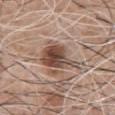Impression: Part of a total-body skin-imaging series; this lesion was reviewed on a skin check and was not flagged for biopsy. Acquisition and patient details: A roughly 15 mm field-of-view crop from a total-body skin photograph. About 4.5 mm across. From the chest. The lesion-visualizer software estimated internal color variation of about 7.5 on a 0–10 scale and peripheral color asymmetry of about 2.5. And it measured an automated nevus-likeness rating near 95 out of 100. A male patient aged 58 to 62.